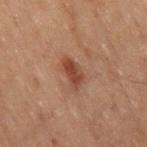Part of a total-body skin-imaging series; this lesion was reviewed on a skin check and was not flagged for biopsy.
The patient is a male roughly 70 years of age.
Approximately 3.5 mm at its widest.
On the right thigh.
The total-body-photography lesion software estimated an area of roughly 5.5 mm², an eccentricity of roughly 0.85, and a shape-asymmetry score of about 0.3 (0 = symmetric). The software also gave an average lesion color of about L≈33 a*≈19 b*≈24 (CIELAB), a lesion–skin lightness drop of about 9, and a normalized border contrast of about 8. And it measured border irregularity of about 3 on a 0–10 scale, internal color variation of about 1.5 on a 0–10 scale, and radial color variation of about 0.5. The analysis additionally found a nevus-likeness score of about 90/100 and lesion-presence confidence of about 100/100.
A roughly 15 mm field-of-view crop from a total-body skin photograph.